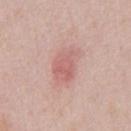Q: Was a biopsy performed?
A: catalogued during a skin exam; not biopsied
Q: What are the patient's age and sex?
A: male, in their 40s
Q: What kind of image is this?
A: ~15 mm crop, total-body skin-cancer survey
Q: What is the anatomic site?
A: the chest
Q: What is the lesion's diameter?
A: about 4.5 mm
Q: Automated lesion metrics?
A: a lesion-to-skin contrast of about 5.5 (normalized; higher = more distinct)